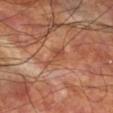Q: Was this lesion biopsied?
A: imaged on a skin check; not biopsied
Q: Where on the body is the lesion?
A: the leg
Q: What are the patient's age and sex?
A: male, about 60 years old
Q: How was this image acquired?
A: 15 mm crop, total-body photography
Q: Illumination type?
A: cross-polarized illumination